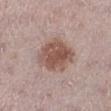{"biopsy_status": "not biopsied; imaged during a skin examination", "site": "right lower leg", "image": {"source": "total-body photography crop", "field_of_view_mm": 15}, "patient": {"sex": "female", "age_approx": 45}}